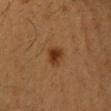biopsy status=no biopsy performed (imaged during a skin exam)
anatomic site=the head or neck
subject=male, aged 38–42
image=~15 mm tile from a whole-body skin photo